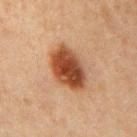  biopsy_status: not biopsied; imaged during a skin examination
  site: mid back
  patient:
    sex: male
    age_approx: 65
  image:
    source: total-body photography crop
    field_of_view_mm: 15
  automated_metrics:
    eccentricity: 0.7
    shape_asymmetry: 0.15
    cielab_L: 41
    cielab_a: 23
    cielab_b: 32
    vs_skin_darker_L: 15.0
  lighting: cross-polarized